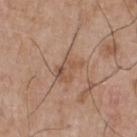{
  "biopsy_status": "not biopsied; imaged during a skin examination",
  "site": "chest",
  "patient": {
    "sex": "male",
    "age_approx": 65
  },
  "image": {
    "source": "total-body photography crop",
    "field_of_view_mm": 15
  },
  "lighting": "white-light",
  "lesion_size": {
    "long_diameter_mm_approx": 4.0
  }
}